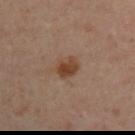Case summary:
• follow-up: imaged on a skin check; not biopsied
• image: 15 mm crop, total-body photography
• patient: male, roughly 45 years of age
• location: the arm
• diameter: about 3 mm